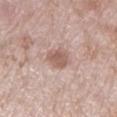{"biopsy_status": "not biopsied; imaged during a skin examination", "lesion_size": {"long_diameter_mm_approx": 2.5}, "patient": {"sex": "female", "age_approx": 75}, "image": {"source": "total-body photography crop", "field_of_view_mm": 15}, "site": "left lower leg", "automated_metrics": {"area_mm2_approx": 5.5, "eccentricity": 0.2, "cielab_L": 58, "cielab_a": 19, "cielab_b": 24, "vs_skin_darker_L": 11.0, "vs_skin_contrast_norm": 7.0, "nevus_likeness_0_100": 50, "lesion_detection_confidence_0_100": 100}, "lighting": "white-light"}